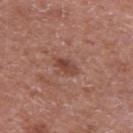Findings:
- workup: total-body-photography surveillance lesion; no biopsy
- illumination: white-light illumination
- body site: the right upper arm
- patient: male, approximately 65 years of age
- imaging modality: 15 mm crop, total-body photography